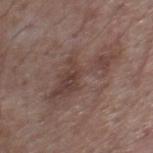The lesion was tiled from a total-body skin photograph and was not biopsied.
Cropped from a whole-body photographic skin survey; the tile spans about 15 mm.
Approximately 8 mm at its widest.
A male subject, in their mid-50s.
From the chest.
The tile uses white-light illumination.